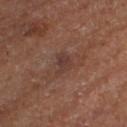Imaged during a routine full-body skin examination; the lesion was not biopsied and no histopathology is available. The patient is female. The lesion is located on the left thigh. A region of skin cropped from a whole-body photographic capture, roughly 15 mm wide. Approximately 3 mm at its widest. This is a cross-polarized tile.